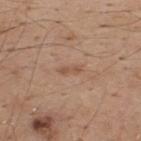Clinical impression: No biopsy was performed on this lesion — it was imaged during a full skin examination and was not determined to be concerning. Acquisition and patient details: The recorded lesion diameter is about 2.5 mm. A roughly 15 mm field-of-view crop from a total-body skin photograph. The lesion is located on the upper back. A male subject, aged approximately 40. The tile uses white-light illumination.